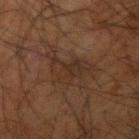{
  "biopsy_status": "not biopsied; imaged during a skin examination",
  "image": {
    "source": "total-body photography crop",
    "field_of_view_mm": 15
  },
  "patient": {
    "sex": "male",
    "age_approx": 65
  },
  "lesion_size": {
    "long_diameter_mm_approx": 6.0
  },
  "site": "right upper arm",
  "automated_metrics": {
    "area_mm2_approx": 11.0,
    "shape_asymmetry": 0.5,
    "cielab_L": 24,
    "cielab_a": 14,
    "cielab_b": 21,
    "vs_skin_darker_L": 5.0,
    "vs_skin_contrast_norm": 5.5,
    "peripheral_color_asymmetry": 1.0
  }
}